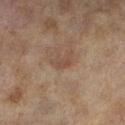Clinical impression:
No biopsy was performed on this lesion — it was imaged during a full skin examination and was not determined to be concerning.
Image and clinical context:
Approximately 3 mm at its widest. Cropped from a whole-body photographic skin survey; the tile spans about 15 mm. Imaged with cross-polarized lighting. A female subject approximately 60 years of age. The lesion is located on the left lower leg. An algorithmic analysis of the crop reported an area of roughly 3.5 mm² and two-axis asymmetry of about 0.4. The analysis additionally found border irregularity of about 4 on a 0–10 scale, a color-variation rating of about 1/10, and peripheral color asymmetry of about 0. And it measured a nevus-likeness score of about 0/100.